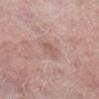Q: What did automated image analysis measure?
A: border irregularity of about 2 on a 0–10 scale, a within-lesion color-variation index near 2/10, and peripheral color asymmetry of about 0.5
Q: Who is the patient?
A: female, approximately 60 years of age
Q: What kind of image is this?
A: ~15 mm crop, total-body skin-cancer survey
Q: How large is the lesion?
A: about 2.5 mm
Q: Where on the body is the lesion?
A: the right lower leg